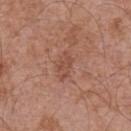Impression:
This lesion was catalogued during total-body skin photography and was not selected for biopsy.
Context:
Located on the chest. Captured under white-light illumination. Cropped from a total-body skin-imaging series; the visible field is about 15 mm. About 3.5 mm across. Automated image analysis of the tile measured an area of roughly 4 mm², an outline eccentricity of about 0.85 (0 = round, 1 = elongated), and a shape-asymmetry score of about 0.55 (0 = symmetric). The software also gave an average lesion color of about L≈49 a*≈23 b*≈29 (CIELAB), roughly 7 lightness units darker than nearby skin, and a lesion-to-skin contrast of about 5.5 (normalized; higher = more distinct). It also reported a nevus-likeness score of about 0/100. A male subject aged around 70.